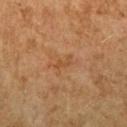patient:
  sex: female
  age_approx: 60
image:
  source: total-body photography crop
  field_of_view_mm: 15
automated_metrics:
  area_mm2_approx: 2.5
  color_variation_0_10: 0.0
  peripheral_color_asymmetry: 0.0
site: arm
lesion_size:
  long_diameter_mm_approx: 2.5
lighting: cross-polarized